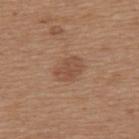follow-up: no biopsy performed (imaged during a skin exam) | image source: ~15 mm crop, total-body skin-cancer survey | subject: female, in their mid-50s | site: the upper back | TBP lesion metrics: an average lesion color of about L≈49 a*≈21 b*≈30 (CIELAB) and a normalized border contrast of about 6; border irregularity of about 1.5 on a 0–10 scale and a peripheral color-asymmetry measure near 1; a detector confidence of about 100 out of 100 that the crop contains a lesion | size: ~3.5 mm (longest diameter).